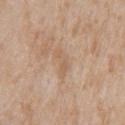Notes:
• notes: catalogued during a skin exam; not biopsied